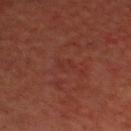biopsy status — imaged on a skin check; not biopsied | patient — male, about 60 years old | body site — the head or neck | TBP lesion metrics — a footprint of about 2.5 mm², an eccentricity of roughly 0.55, and a shape-asymmetry score of about 0.35 (0 = symmetric); a mean CIELAB color near L≈30 a*≈26 b*≈24, roughly 3 lightness units darker than nearby skin, and a lesion-to-skin contrast of about 3 (normalized; higher = more distinct); a border-irregularity index near 3.5/10, internal color variation of about 0.5 on a 0–10 scale, and a peripheral color-asymmetry measure near 0; a nevus-likeness score of about 0/100 and a detector confidence of about 100 out of 100 that the crop contains a lesion | imaging modality — ~15 mm crop, total-body skin-cancer survey | tile lighting — cross-polarized.